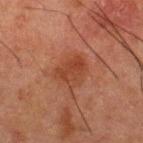Captured during whole-body skin photography for melanoma surveillance; the lesion was not biopsied. Located on the upper back. Longest diameter approximately 3.5 mm. An algorithmic analysis of the crop reported a footprint of about 8 mm² and an outline eccentricity of about 0.6 (0 = round, 1 = elongated). The software also gave a classifier nevus-likeness of about 20/100 and lesion-presence confidence of about 100/100. The patient is a male in their 50s. A roughly 15 mm field-of-view crop from a total-body skin photograph.